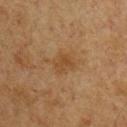{"biopsy_status": "not biopsied; imaged during a skin examination", "image": {"source": "total-body photography crop", "field_of_view_mm": 15}, "lesion_size": {"long_diameter_mm_approx": 2.5}, "patient": {"sex": "male", "age_approx": 65}, "site": "right upper arm", "automated_metrics": {"area_mm2_approx": 5.0, "eccentricity": 0.45, "shape_asymmetry": 0.3, "border_irregularity_0_10": 3.0, "color_variation_0_10": 3.0, "peripheral_color_asymmetry": 1.0, "lesion_detection_confidence_0_100": 100}}